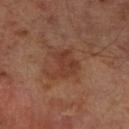Recorded during total-body skin imaging; not selected for excision or biopsy.
This is a cross-polarized tile.
The recorded lesion diameter is about 4 mm.
Automated image analysis of the tile measured an average lesion color of about L≈37 a*≈22 b*≈27 (CIELAB), about 7 CIELAB-L* units darker than the surrounding skin, and a lesion-to-skin contrast of about 6 (normalized; higher = more distinct). It also reported a peripheral color-asymmetry measure near 1. It also reported an automated nevus-likeness rating near 5 out of 100 and lesion-presence confidence of about 100/100.
A 15 mm close-up extracted from a 3D total-body photography capture.
A male subject, in their 70s.
Located on the right lower leg.